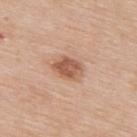workup: imaged on a skin check; not biopsied
site: the upper back
tile lighting: white-light illumination
subject: male, aged approximately 80
imaging modality: ~15 mm tile from a whole-body skin photo
automated lesion analysis: a lesion–skin lightness drop of about 12 and a lesion-to-skin contrast of about 8 (normalized; higher = more distinct)